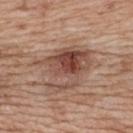workup: catalogued during a skin exam; not biopsied | diameter: about 7 mm | imaging modality: ~15 mm tile from a whole-body skin photo | tile lighting: white-light | anatomic site: the upper back | TBP lesion metrics: a lesion color around L≈48 a*≈21 b*≈26 in CIELAB, about 12 CIELAB-L* units darker than the surrounding skin, and a lesion-to-skin contrast of about 8.5 (normalized; higher = more distinct); a lesion-detection confidence of about 100/100 | patient: female, roughly 40 years of age.